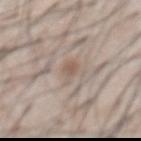No biopsy was performed on this lesion — it was imaged during a full skin examination and was not determined to be concerning.
The subject is a male approximately 60 years of age.
From the abdomen.
Automated tile analysis of the lesion measured an average lesion color of about L≈57 a*≈14 b*≈24 (CIELAB), roughly 8 lightness units darker than nearby skin, and a normalized border contrast of about 6. And it measured a color-variation rating of about 1.5/10 and radial color variation of about 0.5.
A lesion tile, about 15 mm wide, cut from a 3D total-body photograph.
Imaged with white-light lighting.